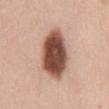Assessment:
Part of a total-body skin-imaging series; this lesion was reviewed on a skin check and was not flagged for biopsy.
Acquisition and patient details:
Cropped from a whole-body photographic skin survey; the tile spans about 15 mm. The total-body-photography lesion software estimated a lesion area of about 21 mm², an eccentricity of roughly 0.8, and a shape-asymmetry score of about 0.15 (0 = symmetric). The patient is a female aged 48 to 52. The tile uses white-light illumination. Approximately 7 mm at its widest. The lesion is located on the chest.